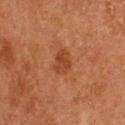This lesion was catalogued during total-body skin photography and was not selected for biopsy.
A female subject about 50 years old.
The lesion is located on the upper back.
A region of skin cropped from a whole-body photographic capture, roughly 15 mm wide.